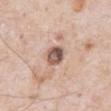workup — no biopsy performed (imaged during a skin exam) | image source — ~15 mm tile from a whole-body skin photo | size — about 2.5 mm | location — the abdomen | subject — male, aged approximately 80.